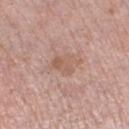  biopsy_status: not biopsied; imaged during a skin examination
  site: left lower leg
  image:
    source: total-body photography crop
    field_of_view_mm: 15
  patient:
    sex: female
    age_approx: 60
  lighting: white-light